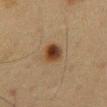Assessment:
The lesion was photographed on a routine skin check and not biopsied; there is no pathology result.
Context:
Cropped from a whole-body photographic skin survey; the tile spans about 15 mm. About 3 mm across. Captured under cross-polarized illumination. On the mid back. The subject is a male aged approximately 60. The lesion-visualizer software estimated a lesion area of about 6 mm², a shape eccentricity near 0.65, and a symmetry-axis asymmetry near 0.2. And it measured a border-irregularity index near 2/10 and a within-lesion color-variation index near 6.5/10. And it measured a nevus-likeness score of about 100/100 and a lesion-detection confidence of about 100/100.